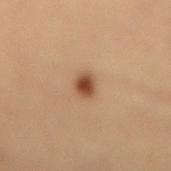| field | value |
|---|---|
| follow-up | total-body-photography surveillance lesion; no biopsy |
| imaging modality | ~15 mm tile from a whole-body skin photo |
| patient | male, roughly 55 years of age |
| location | the lower back |
| diameter | about 2.5 mm |